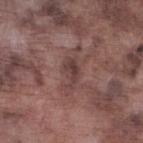Impression: Imaged during a routine full-body skin examination; the lesion was not biopsied and no histopathology is available. Background: A region of skin cropped from a whole-body photographic capture, roughly 15 mm wide. A male subject in their mid- to late 70s. Imaged with white-light lighting. Longest diameter approximately 3 mm. The lesion is on the left lower leg.